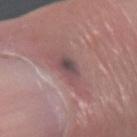The lesion was photographed on a routine skin check and not biopsied; there is no pathology result. The lesion is on the left forearm. A close-up tile cropped from a whole-body skin photograph, about 15 mm across. The patient is a male aged approximately 75.